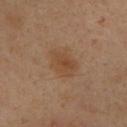follow-up: total-body-photography surveillance lesion; no biopsy | anatomic site: the front of the torso | patient: male, roughly 35 years of age | lesion diameter: ≈4 mm | imaging modality: ~15 mm tile from a whole-body skin photo.